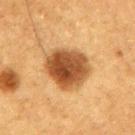A male patient aged 73 to 77.
The lesion-visualizer software estimated a footprint of about 20 mm². And it measured a lesion color around L≈42 a*≈20 b*≈34 in CIELAB, a lesion–skin lightness drop of about 15, and a normalized lesion–skin contrast near 11.5. It also reported border irregularity of about 1.5 on a 0–10 scale, a color-variation rating of about 6/10, and peripheral color asymmetry of about 2. The analysis additionally found a classifier nevus-likeness of about 95/100 and a lesion-detection confidence of about 100/100.
A region of skin cropped from a whole-body photographic capture, roughly 15 mm wide.
The lesion is located on the right upper arm.
Measured at roughly 6 mm in maximum diameter.
Imaged with cross-polarized lighting.